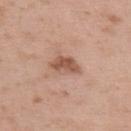biopsy status: catalogued during a skin exam; not biopsied
body site: the upper back
illumination: white-light
acquisition: ~15 mm crop, total-body skin-cancer survey
subject: male, roughly 40 years of age